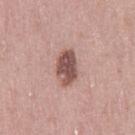Q: Was a biopsy performed?
A: catalogued during a skin exam; not biopsied
Q: Patient demographics?
A: female, approximately 40 years of age
Q: What is the anatomic site?
A: the right thigh
Q: What is the imaging modality?
A: 15 mm crop, total-body photography